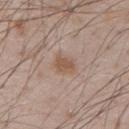Q: Was this lesion biopsied?
A: imaged on a skin check; not biopsied
Q: Where on the body is the lesion?
A: the chest
Q: Patient demographics?
A: male, about 55 years old
Q: What is the imaging modality?
A: 15 mm crop, total-body photography